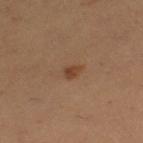This lesion was catalogued during total-body skin photography and was not selected for biopsy. Located on the left thigh. The tile uses cross-polarized illumination. The recorded lesion diameter is about 2 mm. Automated tile analysis of the lesion measured a lesion color around L≈40 a*≈20 b*≈31 in CIELAB, roughly 9 lightness units darker than nearby skin, and a normalized border contrast of about 7.5. A lesion tile, about 15 mm wide, cut from a 3D total-body photograph. A female subject aged approximately 35.